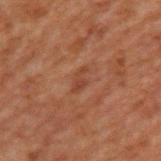This lesion was catalogued during total-body skin photography and was not selected for biopsy. About 3 mm across. On the upper back. The subject is a male aged 68 to 72. A roughly 15 mm field-of-view crop from a total-body skin photograph.